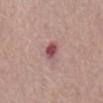Q: Was this lesion biopsied?
A: imaged on a skin check; not biopsied
Q: What is the anatomic site?
A: the abdomen
Q: What are the patient's age and sex?
A: female, aged around 65
Q: What is the imaging modality?
A: 15 mm crop, total-body photography
Q: What did automated image analysis measure?
A: roughly 13 lightness units darker than nearby skin; internal color variation of about 6.5 on a 0–10 scale; a classifier nevus-likeness of about 0/100 and a detector confidence of about 100 out of 100 that the crop contains a lesion